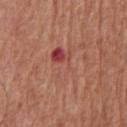{
  "biopsy_status": "not biopsied; imaged during a skin examination",
  "automated_metrics": {
    "area_mm2_approx": 11.0,
    "eccentricity": 0.8,
    "shape_asymmetry": 0.3,
    "cielab_L": 49,
    "cielab_a": 27,
    "cielab_b": 27,
    "vs_skin_darker_L": 6.0,
    "vs_skin_contrast_norm": 4.5,
    "border_irregularity_0_10": 5.0,
    "color_variation_0_10": 10.0,
    "peripheral_color_asymmetry": 5.0,
    "nevus_likeness_0_100": 0,
    "lesion_detection_confidence_0_100": 100
  },
  "image": {
    "source": "total-body photography crop",
    "field_of_view_mm": 15
  },
  "lesion_size": {
    "long_diameter_mm_approx": 5.0
  },
  "site": "chest",
  "patient": {
    "sex": "male",
    "age_approx": 65
  },
  "lighting": "white-light"
}